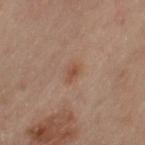Recorded during total-body skin imaging; not selected for excision or biopsy. A female subject in their mid-60s. Imaged with cross-polarized lighting. From the left upper arm. A 15 mm close-up tile from a total-body photography series done for melanoma screening.